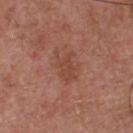{"biopsy_status": "not biopsied; imaged during a skin examination", "patient": {"sex": "male", "age_approx": 55}, "image": {"source": "total-body photography crop", "field_of_view_mm": 15}, "lesion_size": {"long_diameter_mm_approx": 4.0}, "site": "chest", "lighting": "white-light", "automated_metrics": {"cielab_L": 46, "cielab_a": 24, "cielab_b": 29, "vs_skin_darker_L": 6.0, "vs_skin_contrast_norm": 5.5}}